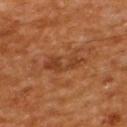Impression: The lesion was tiled from a total-body skin photograph and was not biopsied. Acquisition and patient details: From the upper back. The subject is a male aged approximately 65. Measured at roughly 5 mm in maximum diameter. Captured under cross-polarized illumination. Automated tile analysis of the lesion measured a footprint of about 7 mm² and a shape-asymmetry score of about 0.4 (0 = symmetric). And it measured a within-lesion color-variation index near 2.5/10 and radial color variation of about 0.5. A close-up tile cropped from a whole-body skin photograph, about 15 mm across.